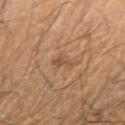{
  "biopsy_status": "not biopsied; imaged during a skin examination",
  "automated_metrics": {
    "border_irregularity_0_10": 5.5,
    "color_variation_0_10": 0.0,
    "peripheral_color_asymmetry": 0.0,
    "lesion_detection_confidence_0_100": 95
  },
  "lesion_size": {
    "long_diameter_mm_approx": 2.5
  },
  "patient": {
    "sex": "male",
    "age_approx": 60
  },
  "lighting": "cross-polarized",
  "site": "right forearm",
  "image": {
    "source": "total-body photography crop",
    "field_of_view_mm": 15
  }
}